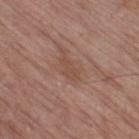Impression:
Recorded during total-body skin imaging; not selected for excision or biopsy.
Background:
A male subject, approximately 70 years of age. Located on the right thigh. Captured under white-light illumination. Longest diameter approximately 3 mm. This image is a 15 mm lesion crop taken from a total-body photograph.